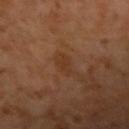Impression: Captured during whole-body skin photography for melanoma surveillance; the lesion was not biopsied. Acquisition and patient details: A male patient in their 60s. A 15 mm close-up tile from a total-body photography series done for melanoma screening. The lesion is on the right forearm. The total-body-photography lesion software estimated border irregularity of about 5 on a 0–10 scale and a peripheral color-asymmetry measure near 0. The analysis additionally found a classifier nevus-likeness of about 0/100.